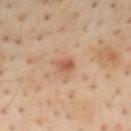Findings:
- biopsy status · catalogued during a skin exam; not biopsied
- lesion size · ≈3 mm
- acquisition · ~15 mm crop, total-body skin-cancer survey
- subject · male, approximately 55 years of age
- body site · the mid back
- illumination · cross-polarized